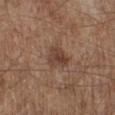workup: no biopsy performed (imaged during a skin exam) | lesion size: ~3 mm (longest diameter) | patient: male, aged 53 to 57 | lighting: white-light | image source: ~15 mm tile from a whole-body skin photo | site: the right lower leg.